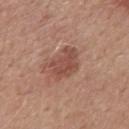The lesion was tiled from a total-body skin photograph and was not biopsied. Automated tile analysis of the lesion measured about 10 CIELAB-L* units darker than the surrounding skin and a lesion-to-skin contrast of about 7 (normalized; higher = more distinct). The patient is a male approximately 50 years of age. This is a white-light tile. Cropped from a whole-body photographic skin survey; the tile spans about 15 mm. The recorded lesion diameter is about 4.5 mm. On the mid back.